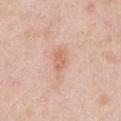biopsy_status: not biopsied; imaged during a skin examination
lesion_size:
  long_diameter_mm_approx: 2.5
image:
  source: total-body photography crop
  field_of_view_mm: 15
patient:
  sex: male
  age_approx: 45
lighting: white-light
site: right upper arm